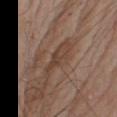Recorded during total-body skin imaging; not selected for excision or biopsy. The lesion is located on the right upper arm. Approximately 5 mm at its widest. A male patient, in their mid-70s. The tile uses white-light illumination. Cropped from a whole-body photographic skin survey; the tile spans about 15 mm.